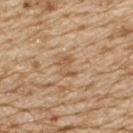– workup · catalogued during a skin exam; not biopsied
– image · ~15 mm tile from a whole-body skin photo
– patient · male, aged around 70
– body site · the upper back